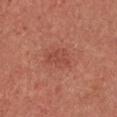This lesion was catalogued during total-body skin photography and was not selected for biopsy. This image is a 15 mm lesion crop taken from a total-body photograph. A female patient aged approximately 35. The lesion is on the upper back.